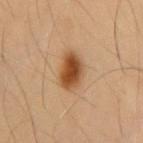<record>
  <biopsy_status>not biopsied; imaged during a skin examination</biopsy_status>
  <lesion_size>
    <long_diameter_mm_approx>4.5</long_diameter_mm_approx>
  </lesion_size>
  <site>mid back</site>
  <lighting>cross-polarized</lighting>
  <image>
    <source>total-body photography crop</source>
    <field_of_view_mm>15</field_of_view_mm>
  </image>
  <patient>
    <sex>male</sex>
    <age_approx>55</age_approx>
  </patient>
  <automated_metrics>
    <area_mm2_approx>9.5</area_mm2_approx>
    <eccentricity>0.8</eccentricity>
    <shape_asymmetry>0.15</shape_asymmetry>
    <cielab_L>48</cielab_L>
    <cielab_a>22</cielab_a>
    <cielab_b>37</cielab_b>
    <vs_skin_darker_L>14.0</vs_skin_darker_L>
    <vs_skin_contrast_norm>10.5</vs_skin_contrast_norm>
    <border_irregularity_0_10>2.0</border_irregularity_0_10>
    <peripheral_color_asymmetry>1.5</peripheral_color_asymmetry>
    <nevus_likeness_0_100>100</nevus_likeness_0_100>
    <lesion_detection_confidence_0_100>100</lesion_detection_confidence_0_100>
  </automated_metrics>
</record>